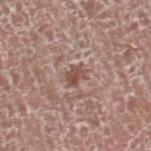Captured under white-light illumination. A male patient roughly 75 years of age. An algorithmic analysis of the crop reported a lesion color around L≈50 a*≈19 b*≈24 in CIELAB, about 10 CIELAB-L* units darker than the surrounding skin, and a lesion-to-skin contrast of about 7.5 (normalized; higher = more distinct). The analysis additionally found lesion-presence confidence of about 90/100. A roughly 15 mm field-of-view crop from a total-body skin photograph. On the right lower leg.